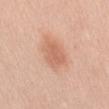{
  "patient": {
    "sex": "female",
    "age_approx": 45
  },
  "image": {
    "source": "total-body photography crop",
    "field_of_view_mm": 15
  },
  "site": "mid back"
}